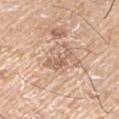Clinical impression:
Imaged during a routine full-body skin examination; the lesion was not biopsied and no histopathology is available.
Context:
A male patient aged around 75. Located on the left upper arm. This is a white-light tile. A lesion tile, about 15 mm wide, cut from a 3D total-body photograph. About 4 mm across. The total-body-photography lesion software estimated a shape eccentricity near 0.9 and a shape-asymmetry score of about 0.7 (0 = symmetric). And it measured an automated nevus-likeness rating near 0 out of 100 and a detector confidence of about 55 out of 100 that the crop contains a lesion.